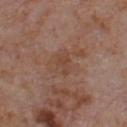Impression: This lesion was catalogued during total-body skin photography and was not selected for biopsy. Image and clinical context: Longest diameter approximately 2.5 mm. Imaged with white-light lighting. A male patient about 65 years old. Cropped from a whole-body photographic skin survey; the tile spans about 15 mm. From the chest.